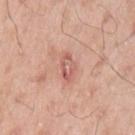The lesion was photographed on a routine skin check and not biopsied; there is no pathology result.
A 15 mm crop from a total-body photograph taken for skin-cancer surveillance.
Measured at roughly 3 mm in maximum diameter.
The lesion is on the left upper arm.
The tile uses white-light illumination.
Automated image analysis of the tile measured a lesion color around L≈59 a*≈25 b*≈27 in CIELAB. And it measured a border-irregularity index near 4/10, a color-variation rating of about 6/10, and a peripheral color-asymmetry measure near 2.5.
The subject is a male aged approximately 55.